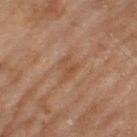The lesion was tiled from a total-body skin photograph and was not biopsied. About 3.5 mm across. A female patient aged around 60. Imaged with cross-polarized lighting. The lesion-visualizer software estimated an eccentricity of roughly 0.7. The software also gave roughly 5 lightness units darker than nearby skin. The analysis additionally found border irregularity of about 4.5 on a 0–10 scale, internal color variation of about 3 on a 0–10 scale, and radial color variation of about 1. From the left lower leg. A region of skin cropped from a whole-body photographic capture, roughly 15 mm wide.